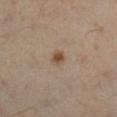Notes:
- workup · total-body-photography surveillance lesion; no biopsy